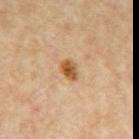Q: Was this lesion biopsied?
A: catalogued during a skin exam; not biopsied
Q: What is the imaging modality?
A: ~15 mm tile from a whole-body skin photo
Q: Automated lesion metrics?
A: a footprint of about 4 mm² and an eccentricity of roughly 0.75; a lesion–skin lightness drop of about 13; a within-lesion color-variation index near 3.5/10
Q: Where on the body is the lesion?
A: the upper back
Q: What are the patient's age and sex?
A: male, about 85 years old or older
Q: How large is the lesion?
A: ~2.5 mm (longest diameter)